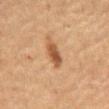Q: Lesion location?
A: the mid back
Q: What are the patient's age and sex?
A: male, roughly 65 years of age
Q: What kind of image is this?
A: 15 mm crop, total-body photography